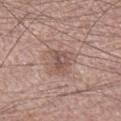workup=catalogued during a skin exam; not biopsied | anatomic site=the right lower leg | patient=male, aged 53–57 | diameter=~3 mm (longest diameter) | image source=15 mm crop, total-body photography | illumination=white-light.